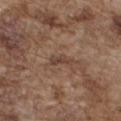Assessment:
Part of a total-body skin-imaging series; this lesion was reviewed on a skin check and was not flagged for biopsy.
Image and clinical context:
The total-body-photography lesion software estimated a lesion color around L≈42 a*≈19 b*≈25 in CIELAB and a lesion–skin lightness drop of about 8. The analysis additionally found an automated nevus-likeness rating near 0 out of 100 and lesion-presence confidence of about 95/100. Cropped from a total-body skin-imaging series; the visible field is about 15 mm. The lesion is on the chest. The patient is a male in their mid- to late 70s. Imaged with white-light lighting.